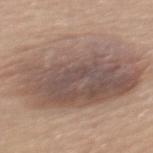This is a white-light tile.
The lesion is located on the mid back.
The patient is a female aged around 65.
A roughly 15 mm field-of-view crop from a total-body skin photograph.